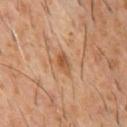Assessment:
Captured during whole-body skin photography for melanoma surveillance; the lesion was not biopsied.
Acquisition and patient details:
The subject is a male aged 58 to 62. The recorded lesion diameter is about 3 mm. Located on the chest. Cropped from a whole-body photographic skin survey; the tile spans about 15 mm.